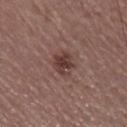{"biopsy_status": "not biopsied; imaged during a skin examination", "patient": {"sex": "male", "age_approx": 65}, "image": {"source": "total-body photography crop", "field_of_view_mm": 15}, "site": "right lower leg", "lighting": "white-light", "lesion_size": {"long_diameter_mm_approx": 2.5}}